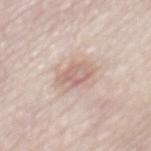Impression: No biopsy was performed on this lesion — it was imaged during a full skin examination and was not determined to be concerning.